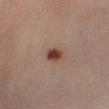Q: Was this lesion biopsied?
A: catalogued during a skin exam; not biopsied
Q: Where on the body is the lesion?
A: the left lower leg
Q: What is the imaging modality?
A: 15 mm crop, total-body photography
Q: Who is the patient?
A: female, aged around 50